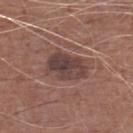Q: Was a biopsy performed?
A: total-body-photography surveillance lesion; no biopsy
Q: What is the anatomic site?
A: the left lower leg
Q: How was this image acquired?
A: ~15 mm crop, total-body skin-cancer survey
Q: What are the patient's age and sex?
A: male, aged 73–77